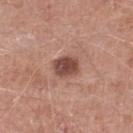A male subject aged around 60. This is a white-light tile. A 15 mm close-up extracted from a 3D total-body photography capture. Longest diameter approximately 3 mm. The total-body-photography lesion software estimated an outline eccentricity of about 0.6 (0 = round, 1 = elongated) and two-axis asymmetry of about 0.2. From the right lower leg.